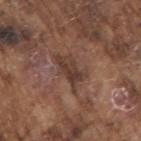| key | value |
|---|---|
| notes | catalogued during a skin exam; not biopsied |
| subject | male, in their mid-70s |
| lighting | white-light illumination |
| TBP lesion metrics | roughly 9 lightness units darker than nearby skin and a normalized border contrast of about 8; internal color variation of about 4.5 on a 0–10 scale; a classifier nevus-likeness of about 0/100 and a lesion-detection confidence of about 65/100 |
| size | about 4.5 mm |
| body site | the right upper arm |
| image source | ~15 mm crop, total-body skin-cancer survey |